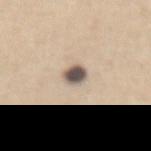Clinical impression:
Recorded during total-body skin imaging; not selected for excision or biopsy.
Background:
On the chest. A female patient, aged 58 to 62. Captured under white-light illumination. A 15 mm close-up tile from a total-body photography series done for melanoma screening.